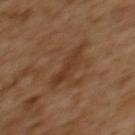{"site": "back", "lesion_size": {"long_diameter_mm_approx": 4.5}, "automated_metrics": {"area_mm2_approx": 7.0, "eccentricity": 0.9, "shape_asymmetry": 0.35, "border_irregularity_0_10": 4.5, "nevus_likeness_0_100": 0, "lesion_detection_confidence_0_100": 100}, "lighting": "cross-polarized", "image": {"source": "total-body photography crop", "field_of_view_mm": 15}, "patient": {"sex": "female", "age_approx": 55}}